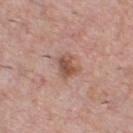Q: Was a biopsy performed?
A: imaged on a skin check; not biopsied
Q: What kind of image is this?
A: ~15 mm tile from a whole-body skin photo
Q: Where on the body is the lesion?
A: the chest
Q: What are the patient's age and sex?
A: male, aged around 40